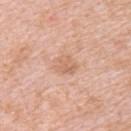Imaged during a routine full-body skin examination; the lesion was not biopsied and no histopathology is available.
This is a white-light tile.
The lesion's longest dimension is about 3 mm.
Located on the left upper arm.
A female subject, roughly 45 years of age.
A 15 mm crop from a total-body photograph taken for skin-cancer surveillance.
An algorithmic analysis of the crop reported an area of roughly 5.5 mm² and two-axis asymmetry of about 0.25. The software also gave a nevus-likeness score of about 0/100 and a detector confidence of about 100 out of 100 that the crop contains a lesion.